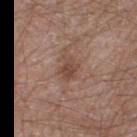follow-up = no biopsy performed (imaged during a skin exam).